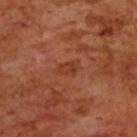The lesion was photographed on a routine skin check and not biopsied; there is no pathology result. The patient is a male aged 68–72. The lesion is located on the back. A 15 mm close-up extracted from a 3D total-body photography capture.